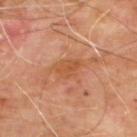biopsy status: imaged on a skin check; not biopsied | imaging modality: total-body-photography crop, ~15 mm field of view | automated metrics: a lesion area of about 5.5 mm², an eccentricity of roughly 0.75, and two-axis asymmetry of about 0.3; a mean CIELAB color near L≈51 a*≈25 b*≈37 and a lesion-to-skin contrast of about 6.5 (normalized; higher = more distinct); a nevus-likeness score of about 0/100 and lesion-presence confidence of about 100/100 | diameter: about 3.5 mm | location: the upper back | subject: male, aged approximately 65.